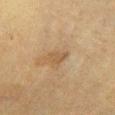biopsy status=imaged on a skin check; not biopsied
patient=male, about 60 years old
tile lighting=cross-polarized illumination
diameter=about 3 mm
body site=the leg
image source=~15 mm tile from a whole-body skin photo